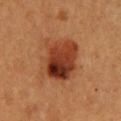Impression:
Imaged during a routine full-body skin examination; the lesion was not biopsied and no histopathology is available.
Context:
A female subject approximately 60 years of age. Cropped from a whole-body photographic skin survey; the tile spans about 15 mm. The lesion is located on the upper back.